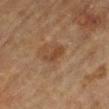The lesion was photographed on a routine skin check and not biopsied; there is no pathology result. About 3 mm across. Cropped from a total-body skin-imaging series; the visible field is about 15 mm. A male subject aged approximately 85 or older. From the left lower leg. The lesion-visualizer software estimated an average lesion color of about L≈37 a*≈18 b*≈29 (CIELAB), roughly 7 lightness units darker than nearby skin, and a normalized lesion–skin contrast near 6.5. It also reported border irregularity of about 4.5 on a 0–10 scale. And it measured a classifier nevus-likeness of about 10/100 and a detector confidence of about 100 out of 100 that the crop contains a lesion. The tile uses cross-polarized illumination.